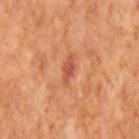Part of a total-body skin-imaging series; this lesion was reviewed on a skin check and was not flagged for biopsy. A male subject, in their mid- to late 60s. Cropped from a total-body skin-imaging series; the visible field is about 15 mm.